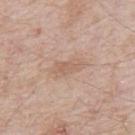Clinical impression:
Recorded during total-body skin imaging; not selected for excision or biopsy.
Clinical summary:
An algorithmic analysis of the crop reported an average lesion color of about L≈61 a*≈18 b*≈28 (CIELAB), roughly 7 lightness units darker than nearby skin, and a normalized border contrast of about 5. It also reported a border-irregularity index near 3.5/10 and peripheral color asymmetry of about 0.5. On the mid back. The recorded lesion diameter is about 4 mm. A male patient roughly 65 years of age. A 15 mm crop from a total-body photograph taken for skin-cancer surveillance. The tile uses white-light illumination.